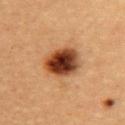Clinical impression: Recorded during total-body skin imaging; not selected for excision or biopsy. Image and clinical context: A female patient, aged around 30. The recorded lesion diameter is about 4.5 mm. An algorithmic analysis of the crop reported a lesion area of about 13 mm², a shape eccentricity near 0.7, and a symmetry-axis asymmetry near 0.2. And it measured a lesion–skin lightness drop of about 20 and a lesion-to-skin contrast of about 15 (normalized; higher = more distinct). It also reported an automated nevus-likeness rating near 100 out of 100. A lesion tile, about 15 mm wide, cut from a 3D total-body photograph. On the back. Captured under cross-polarized illumination.